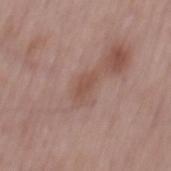Part of a total-body skin-imaging series; this lesion was reviewed on a skin check and was not flagged for biopsy.
Cropped from a whole-body photographic skin survey; the tile spans about 15 mm.
The lesion's longest dimension is about 2.5 mm.
The subject is a male aged 53–57.
Automated image analysis of the tile measured a lesion area of about 3 mm² and an eccentricity of roughly 0.85. It also reported a border-irregularity index near 3/10, a within-lesion color-variation index near 1/10, and radial color variation of about 0.5.
The tile uses white-light illumination.